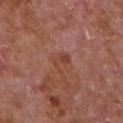The lesion was photographed on a routine skin check and not biopsied; there is no pathology result. The tile uses white-light illumination. A 15 mm close-up extracted from a 3D total-body photography capture. A male patient, roughly 65 years of age. From the front of the torso.